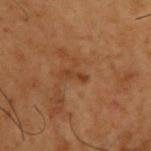Part of a total-body skin-imaging series; this lesion was reviewed on a skin check and was not flagged for biopsy. Captured under cross-polarized illumination. The recorded lesion diameter is about 2.5 mm. The lesion is on the back. A 15 mm crop from a total-body photograph taken for skin-cancer surveillance. A male patient in their mid-50s.